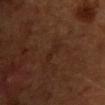No biopsy was performed on this lesion — it was imaged during a full skin examination and was not determined to be concerning. Located on the front of the torso. Measured at roughly 2.5 mm in maximum diameter. A 15 mm close-up extracted from a 3D total-body photography capture. This is a cross-polarized tile. A male patient, aged approximately 60.